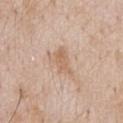Notes:
* notes — no biopsy performed (imaged during a skin exam)
* site — the chest
* imaging modality — ~15 mm crop, total-body skin-cancer survey
* illumination — white-light
* subject — male, roughly 60 years of age
* lesion size — ≈4 mm